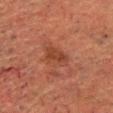Q: What kind of image is this?
A: 15 mm crop, total-body photography
Q: How was the tile lit?
A: cross-polarized illumination
Q: Patient demographics?
A: male, aged 58–62
Q: How large is the lesion?
A: ~3 mm (longest diameter)
Q: Where on the body is the lesion?
A: the head or neck